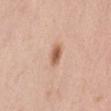follow-up: catalogued during a skin exam; not biopsied
image source: total-body-photography crop, ~15 mm field of view
diameter: ≈2.5 mm
tile lighting: white-light
subject: female, aged 48–52
automated lesion analysis: a shape eccentricity near 0.75 and a symmetry-axis asymmetry near 0.15; a mean CIELAB color near L≈60 a*≈22 b*≈33, a lesion–skin lightness drop of about 13, and a lesion-to-skin contrast of about 8.5 (normalized; higher = more distinct); a border-irregularity index near 1.5/10 and peripheral color asymmetry of about 1
location: the abdomen